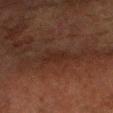Clinical impression:
The lesion was tiled from a total-body skin photograph and was not biopsied.
Clinical summary:
A close-up tile cropped from a whole-body skin photograph, about 15 mm across. Longest diameter approximately 2.5 mm. Imaged with cross-polarized lighting. On the right forearm. A male patient roughly 75 years of age.